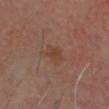Clinical impression:
Recorded during total-body skin imaging; not selected for excision or biopsy.